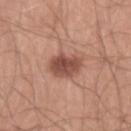image source: ~15 mm crop, total-body skin-cancer survey | anatomic site: the right lower leg | patient: male, aged 38 to 42.